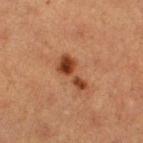Recorded during total-body skin imaging; not selected for excision or biopsy.
The lesion is located on the leg.
A 15 mm close-up extracted from a 3D total-body photography capture.
Captured under cross-polarized illumination.
Longest diameter approximately 4.5 mm.
A female patient approximately 40 years of age.